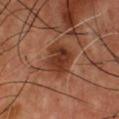<case>
  <biopsy_status>not biopsied; imaged during a skin examination</biopsy_status>
  <lesion_size>
    <long_diameter_mm_approx>4.5</long_diameter_mm_approx>
  </lesion_size>
  <image>
    <source>total-body photography crop</source>
    <field_of_view_mm>15</field_of_view_mm>
  </image>
  <patient>
    <sex>male</sex>
    <age_approx>55</age_approx>
  </patient>
  <site>chest</site>
  <lighting>cross-polarized</lighting>
</case>